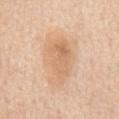Q: Was this lesion biopsied?
A: imaged on a skin check; not biopsied
Q: How was this image acquired?
A: 15 mm crop, total-body photography
Q: Patient demographics?
A: female, in their mid-60s
Q: Lesion location?
A: the mid back
Q: What lighting was used for the tile?
A: white-light